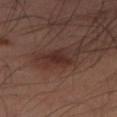The lesion was photographed on a routine skin check and not biopsied; there is no pathology result. A male patient, approximately 50 years of age. This is a cross-polarized tile. On the right thigh. Cropped from a total-body skin-imaging series; the visible field is about 15 mm. Automated tile analysis of the lesion measured a lesion area of about 5 mm², an eccentricity of roughly 0.85, and a shape-asymmetry score of about 0.3 (0 = symmetric). It also reported a lesion color around L≈26 a*≈18 b*≈20 in CIELAB and a normalized lesion–skin contrast near 8. The software also gave a border-irregularity index near 3/10, a within-lesion color-variation index near 1.5/10, and a peripheral color-asymmetry measure near 0.5. The analysis additionally found a detector confidence of about 100 out of 100 that the crop contains a lesion. Approximately 3.5 mm at its widest.